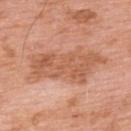Case summary:
* notes — total-body-photography surveillance lesion; no biopsy
* tile lighting — white-light illumination
* image source — ~15 mm tile from a whole-body skin photo
* site — the back
* patient — male, aged around 60
* lesion size — ≈9 mm